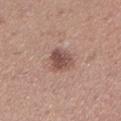This lesion was catalogued during total-body skin photography and was not selected for biopsy. A roughly 15 mm field-of-view crop from a total-body skin photograph. Imaged with white-light lighting. Longest diameter approximately 3 mm. On the left lower leg. A female patient, aged 28–32. The total-body-photography lesion software estimated a mean CIELAB color near L≈50 a*≈20 b*≈24, roughly 12 lightness units darker than nearby skin, and a normalized lesion–skin contrast near 8.5. And it measured a within-lesion color-variation index near 4/10 and peripheral color asymmetry of about 1.5.